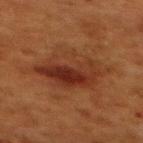The lesion was photographed on a routine skin check and not biopsied; there is no pathology result. Located on the upper back. A female subject, aged around 50. Imaged with cross-polarized lighting. Cropped from a whole-body photographic skin survey; the tile spans about 15 mm. Longest diameter approximately 7.5 mm.